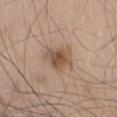notes — imaged on a skin check; not biopsied
lesion size — about 3.5 mm
location — the left thigh
image-analysis metrics — an eccentricity of roughly 0.45; border irregularity of about 3 on a 0–10 scale, a color-variation rating of about 5.5/10, and radial color variation of about 1.5
patient — male, about 40 years old
acquisition — ~15 mm crop, total-body skin-cancer survey
lighting — white-light